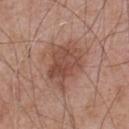notes: catalogued during a skin exam; not biopsied | patient: male, aged around 65 | image source: total-body-photography crop, ~15 mm field of view | location: the upper back | tile lighting: white-light.